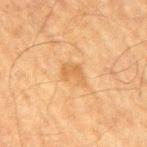| feature | finding |
|---|---|
| workup | total-body-photography surveillance lesion; no biopsy |
| acquisition | ~15 mm tile from a whole-body skin photo |
| site | the right upper arm |
| patient | male, in their mid-60s |